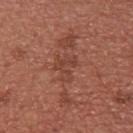Clinical impression:
Imaged during a routine full-body skin examination; the lesion was not biopsied and no histopathology is available.
Acquisition and patient details:
The tile uses white-light illumination. Cropped from a total-body skin-imaging series; the visible field is about 15 mm. About 3.5 mm across. The lesion is on the upper back. A female patient about 25 years old.